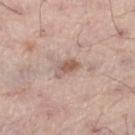Impression: No biopsy was performed on this lesion — it was imaged during a full skin examination and was not determined to be concerning. Background: A 15 mm close-up extracted from a 3D total-body photography capture. The lesion is on the left thigh. A male subject, in their mid-50s.